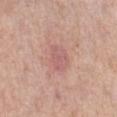The lesion was tiled from a total-body skin photograph and was not biopsied.
Cropped from a whole-body photographic skin survey; the tile spans about 15 mm.
From the leg.
A female subject aged 63–67.
About 3.5 mm across.